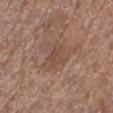Captured during whole-body skin photography for melanoma surveillance; the lesion was not biopsied. Longest diameter approximately 3 mm. A 15 mm close-up extracted from a 3D total-body photography capture. The lesion-visualizer software estimated an area of roughly 4.5 mm², an eccentricity of roughly 0.65, and two-axis asymmetry of about 0.3. It also reported a lesion–skin lightness drop of about 6 and a normalized lesion–skin contrast near 4.5. It also reported a nevus-likeness score of about 0/100. Imaged with white-light lighting. The lesion is on the right lower leg. A female patient aged around 80.